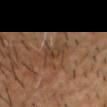Clinical impression:
Part of a total-body skin-imaging series; this lesion was reviewed on a skin check and was not flagged for biopsy.
Clinical summary:
Automated image analysis of the tile measured a lesion area of about 6.5 mm², a shape eccentricity near 0.8, and two-axis asymmetry of about 0.45. The analysis additionally found about 6 CIELAB-L* units darker than the surrounding skin and a lesion-to-skin contrast of about 5.5 (normalized; higher = more distinct). It also reported a border-irregularity rating of about 5.5/10, a color-variation rating of about 4/10, and radial color variation of about 1.5. This is a cross-polarized tile. On the head or neck. Longest diameter approximately 4 mm. The subject is a male roughly 50 years of age. This image is a 15 mm lesion crop taken from a total-body photograph.